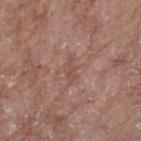| feature | finding |
|---|---|
| biopsy status | total-body-photography surveillance lesion; no biopsy |
| automated lesion analysis | a footprint of about 3.5 mm², an eccentricity of roughly 0.9, and a symmetry-axis asymmetry near 0.3 |
| tile lighting | white-light illumination |
| imaging modality | ~15 mm tile from a whole-body skin photo |
| body site | the leg |
| patient | female, approximately 75 years of age |